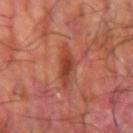The lesion was tiled from a total-body skin photograph and was not biopsied. On the left forearm. The patient is a male approximately 60 years of age. The total-body-photography lesion software estimated an area of roughly 7 mm² and a shape eccentricity near 0.9. And it measured a lesion–skin lightness drop of about 9 and a normalized border contrast of about 7. Measured at roughly 5 mm in maximum diameter. A 15 mm close-up tile from a total-body photography series done for melanoma screening.